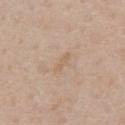workup: no biopsy performed (imaged during a skin exam)
location: the front of the torso
size: ~3 mm (longest diameter)
automated metrics: a lesion-to-skin contrast of about 4.5 (normalized; higher = more distinct)
patient: male, aged approximately 55
lighting: white-light illumination
acquisition: 15 mm crop, total-body photography